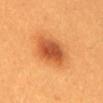follow-up: total-body-photography surveillance lesion; no biopsy
lesion diameter: ≈4.5 mm
automated lesion analysis: a lesion area of about 14 mm² and an outline eccentricity of about 0.55 (0 = round, 1 = elongated)
patient: female, about 30 years old
imaging modality: ~15 mm tile from a whole-body skin photo
site: the abdomen